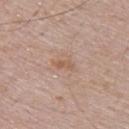Impression: The lesion was tiled from a total-body skin photograph and was not biopsied. Background: A male patient aged 48–52. The recorded lesion diameter is about 2.5 mm. From the upper back. A region of skin cropped from a whole-body photographic capture, roughly 15 mm wide.